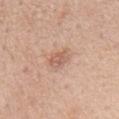Recorded during total-body skin imaging; not selected for excision or biopsy. Longest diameter approximately 2.5 mm. From the mid back. The tile uses white-light illumination. A close-up tile cropped from a whole-body skin photograph, about 15 mm across. A male patient, aged 68–72.